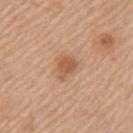follow-up=total-body-photography surveillance lesion; no biopsy | lighting=white-light | lesion diameter=about 3 mm | subject=female, aged approximately 60 | location=the arm | image=15 mm crop, total-body photography.